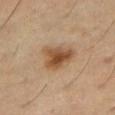The lesion was photographed on a routine skin check and not biopsied; there is no pathology result. The subject is a male about 60 years old. The lesion-visualizer software estimated a lesion area of about 9.5 mm², a shape eccentricity near 0.65, and a symmetry-axis asymmetry near 0.35. The analysis additionally found radial color variation of about 1.5. The analysis additionally found a nevus-likeness score of about 95/100 and lesion-presence confidence of about 100/100. This image is a 15 mm lesion crop taken from a total-body photograph. Longest diameter approximately 4 mm. The lesion is located on the right thigh. Imaged with cross-polarized lighting.